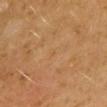Notes:
* acquisition: 15 mm crop, total-body photography
* lighting: cross-polarized illumination
* lesion size: about 1 mm
* location: the mid back
* patient: male, aged around 60